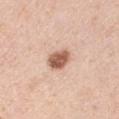biopsy status: catalogued during a skin exam; not biopsied
automated metrics: a lesion area of about 6 mm², an outline eccentricity of about 0.7 (0 = round, 1 = elongated), and a shape-asymmetry score of about 0.15 (0 = symmetric); an average lesion color of about L≈59 a*≈22 b*≈30 (CIELAB) and a normalized border contrast of about 10; a border-irregularity rating of about 1.5/10, internal color variation of about 4 on a 0–10 scale, and peripheral color asymmetry of about 1.5; an automated nevus-likeness rating near 95 out of 100
imaging modality: ~15 mm crop, total-body skin-cancer survey
patient: male, aged around 60
lesion diameter: ≈3.5 mm
anatomic site: the right upper arm
lighting: white-light illumination